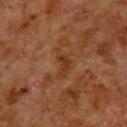{"biopsy_status": "not biopsied; imaged during a skin examination", "lighting": "cross-polarized", "patient": {"sex": "male", "age_approx": 80}, "site": "back", "image": {"source": "total-body photography crop", "field_of_view_mm": 15}}